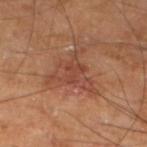| feature | finding |
|---|---|
| follow-up | imaged on a skin check; not biopsied |
| anatomic site | the left lower leg |
| tile lighting | cross-polarized illumination |
| patient | male, aged 63 to 67 |
| image source | ~15 mm tile from a whole-body skin photo |
| diameter | about 5.5 mm |
| automated lesion analysis | a lesion area of about 12 mm² and a shape-asymmetry score of about 0.55 (0 = symmetric); an average lesion color of about L≈46 a*≈25 b*≈30 (CIELAB) and a lesion–skin lightness drop of about 8; a border-irregularity rating of about 8/10, internal color variation of about 3.5 on a 0–10 scale, and radial color variation of about 1 |